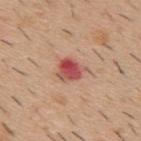imaging modality=total-body-photography crop, ~15 mm field of view; body site=the mid back; tile lighting=white-light illumination; size=≈3.5 mm; subject=male, roughly 40 years of age.